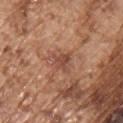body site = the chest | imaging modality = total-body-photography crop, ~15 mm field of view | patient = male, aged around 75 | lighting = white-light illumination | diameter = ~2.5 mm (longest diameter) | automated lesion analysis = an area of roughly 4.5 mm²; a lesion-detection confidence of about 100/100.